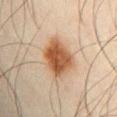{"biopsy_status": "not biopsied; imaged during a skin examination", "image": {"source": "total-body photography crop", "field_of_view_mm": 15}, "patient": {"sex": "male", "age_approx": 45}, "site": "abdomen", "lesion_size": {"long_diameter_mm_approx": 5.5}}